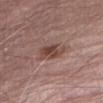Assessment: Imaged during a routine full-body skin examination; the lesion was not biopsied and no histopathology is available. Image and clinical context: Cropped from a total-body skin-imaging series; the visible field is about 15 mm. A male patient, aged around 80. About 3.5 mm across. The total-body-photography lesion software estimated a footprint of about 6.5 mm², an eccentricity of roughly 0.8, and a shape-asymmetry score of about 0.3 (0 = symmetric). The analysis additionally found a classifier nevus-likeness of about 70/100. The tile uses white-light illumination. On the leg.